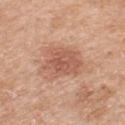The lesion was tiled from a total-body skin photograph and was not biopsied. This is a white-light tile. A 15 mm crop from a total-body photograph taken for skin-cancer surveillance. The lesion is located on the upper back. The patient is a male in their 60s.